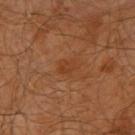automated_metrics:
  vs_skin_darker_L: 5.0
  border_irregularity_0_10: 3.0
  color_variation_0_10: 2.0
  peripheral_color_asymmetry: 1.0
patient:
  sex: male
  age_approx: 65
lighting: cross-polarized
image:
  source: total-body photography crop
  field_of_view_mm: 15
site: right upper arm